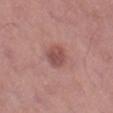No biopsy was performed on this lesion — it was imaged during a full skin examination and was not determined to be concerning.
About 3 mm across.
The subject is a male in their mid-50s.
The lesion is located on the left thigh.
This is a white-light tile.
A 15 mm crop from a total-body photograph taken for skin-cancer surveillance.